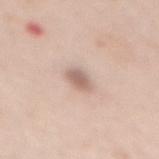site = the back
patient = female, aged 58 to 62
image = 15 mm crop, total-body photography
diameter = about 2.5 mm